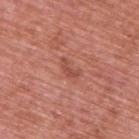biopsy_status: not biopsied; imaged during a skin examination
patient:
  sex: male
  age_approx: 70
site: upper back
lighting: white-light
image:
  source: total-body photography crop
  field_of_view_mm: 15
lesion_size:
  long_diameter_mm_approx: 2.5